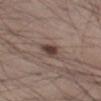Imaged during a routine full-body skin examination; the lesion was not biopsied and no histopathology is available. Cropped from a whole-body photographic skin survey; the tile spans about 15 mm. From the leg. A male subject in their mid-50s.